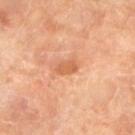The lesion was photographed on a routine skin check and not biopsied; there is no pathology result.
About 2.5 mm across.
Located on the left lower leg.
The patient is a female roughly 70 years of age.
A 15 mm close-up extracted from a 3D total-body photography capture.